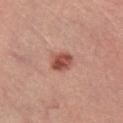site=the right lower leg
TBP lesion metrics=an average lesion color of about L≈46 a*≈25 b*≈27 (CIELAB) and roughly 12 lightness units darker than nearby skin; a border-irregularity index near 1.5/10, a within-lesion color-variation index near 4/10, and peripheral color asymmetry of about 1.5
tile lighting=cross-polarized
subject=male, aged 58 to 62
imaging modality=15 mm crop, total-body photography